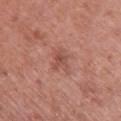Clinical impression:
Captured during whole-body skin photography for melanoma surveillance; the lesion was not biopsied.
Clinical summary:
From the upper back. Automated tile analysis of the lesion measured a mean CIELAB color near L≈51 a*≈25 b*≈27, about 8 CIELAB-L* units darker than the surrounding skin, and a normalized border contrast of about 5.5. The analysis additionally found border irregularity of about 4 on a 0–10 scale, internal color variation of about 3 on a 0–10 scale, and radial color variation of about 1. And it measured a nevus-likeness score of about 0/100 and a lesion-detection confidence of about 100/100. Imaged with white-light lighting. A female subject, roughly 60 years of age. A close-up tile cropped from a whole-body skin photograph, about 15 mm across.